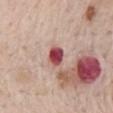Captured during whole-body skin photography for melanoma surveillance; the lesion was not biopsied.
A 15 mm close-up extracted from a 3D total-body photography capture.
The lesion is on the mid back.
This is a white-light tile.
A male patient, about 65 years old.
Automated image analysis of the tile measured a within-lesion color-variation index near 7.5/10. It also reported an automated nevus-likeness rating near 0 out of 100 and a detector confidence of about 100 out of 100 that the crop contains a lesion.